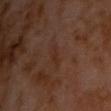biopsy_status: not biopsied; imaged during a skin examination
site: chest
automated_metrics:
  area_mm2_approx: 2.5
  eccentricity: 0.9
  shape_asymmetry: 0.35
  cielab_L: 26
  cielab_a: 17
  cielab_b: 23
  vs_skin_darker_L: 4.0
  vs_skin_contrast_norm: 5.0
image:
  source: total-body photography crop
  field_of_view_mm: 15
patient:
  sex: male
  age_approx: 60
lighting: cross-polarized
lesion_size:
  long_diameter_mm_approx: 2.5